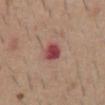workup = no biopsy performed (imaged during a skin exam) | lesion size = ≈2.5 mm | imaging modality = ~15 mm tile from a whole-body skin photo | patient = male, aged 58–62 | illumination = white-light illumination | body site = the abdomen.